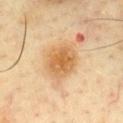Imaged during a routine full-body skin examination; the lesion was not biopsied and no histopathology is available. From the chest. The lesion-visualizer software estimated an area of roughly 13 mm² and a shape-asymmetry score of about 0.15 (0 = symmetric). The analysis additionally found a lesion color around L≈65 a*≈21 b*≈43 in CIELAB and a lesion-to-skin contrast of about 8 (normalized; higher = more distinct). And it measured a nevus-likeness score of about 75/100. A male patient, aged 53–57. Approximately 4.5 mm at its widest. A 15 mm close-up tile from a total-body photography series done for melanoma screening.